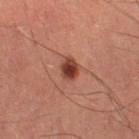Clinical impression: The lesion was tiled from a total-body skin photograph and was not biopsied. Context: A close-up tile cropped from a whole-body skin photograph, about 15 mm across. A male subject, approximately 60 years of age. This is a cross-polarized tile. On the leg.